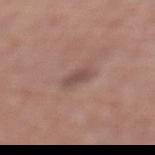No biopsy was performed on this lesion — it was imaged during a full skin examination and was not determined to be concerning. A 15 mm close-up tile from a total-body photography series done for melanoma screening. A female subject aged around 75. The total-body-photography lesion software estimated about 9 CIELAB-L* units darker than the surrounding skin and a normalized lesion–skin contrast near 6.5. And it measured a detector confidence of about 100 out of 100 that the crop contains a lesion. Located on the left lower leg.